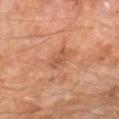| key | value |
|---|---|
| notes | catalogued during a skin exam; not biopsied |
| lesion size | ≈2.5 mm |
| subject | male, aged approximately 60 |
| image source | 15 mm crop, total-body photography |
| anatomic site | the leg |